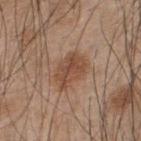Clinical impression:
Recorded during total-body skin imaging; not selected for excision or biopsy.
Clinical summary:
The lesion is on the upper back. Longest diameter approximately 4.5 mm. Imaged with white-light lighting. The subject is a male roughly 45 years of age. Cropped from a total-body skin-imaging series; the visible field is about 15 mm. The lesion-visualizer software estimated an area of roughly 10 mm², an eccentricity of roughly 0.75, and a symmetry-axis asymmetry near 0.2. The analysis additionally found a border-irregularity index near 2.5/10, a within-lesion color-variation index near 4/10, and radial color variation of about 1.5. It also reported a classifier nevus-likeness of about 30/100 and lesion-presence confidence of about 100/100.